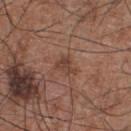This lesion was catalogued during total-body skin photography and was not selected for biopsy. The subject is a male in their mid-50s. This image is a 15 mm lesion crop taken from a total-body photograph. From the chest.